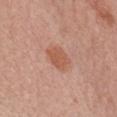Clinical impression: This lesion was catalogued during total-body skin photography and was not selected for biopsy. Background: Located on the chest. This is a white-light tile. The lesion-visualizer software estimated an area of roughly 6 mm² and a shape-asymmetry score of about 0.2 (0 = symmetric). It also reported an average lesion color of about L≈56 a*≈25 b*≈32 (CIELAB) and roughly 8 lightness units darker than nearby skin. And it measured a border-irregularity index near 2/10, a within-lesion color-variation index near 2/10, and a peripheral color-asymmetry measure near 0.5. This image is a 15 mm lesion crop taken from a total-body photograph. A female patient about 50 years old.